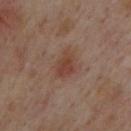Findings:
– workup — catalogued during a skin exam; not biopsied
– automated metrics — a mean CIELAB color near L≈39 a*≈20 b*≈25, roughly 7 lightness units darker than nearby skin, and a normalized border contrast of about 7; border irregularity of about 2.5 on a 0–10 scale, a within-lesion color-variation index near 2.5/10, and peripheral color asymmetry of about 1
– lesion size — about 3 mm
– subject — male, in their mid- to late 40s
– site — the upper back
– acquisition — 15 mm crop, total-body photography
– tile lighting — cross-polarized illumination